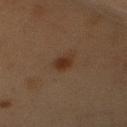Measured at roughly 2.5 mm in maximum diameter.
From the mid back.
The lesion-visualizer software estimated a lesion area of about 3.5 mm², an outline eccentricity of about 0.8 (0 = round, 1 = elongated), and a shape-asymmetry score of about 0.15 (0 = symmetric). The analysis additionally found a mean CIELAB color near L≈26 a*≈15 b*≈25 and a normalized border contrast of about 9.
The patient is a female in their 40s.
A region of skin cropped from a whole-body photographic capture, roughly 15 mm wide.
This is a cross-polarized tile.